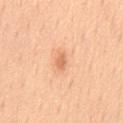Q: Was a biopsy performed?
A: catalogued during a skin exam; not biopsied
Q: Lesion size?
A: ~2.5 mm (longest diameter)
Q: What are the patient's age and sex?
A: male, aged 48–52
Q: What kind of image is this?
A: ~15 mm crop, total-body skin-cancer survey
Q: How was the tile lit?
A: white-light illumination
Q: Where on the body is the lesion?
A: the front of the torso